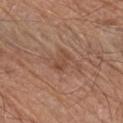image source: ~15 mm crop, total-body skin-cancer survey; body site: the arm; subject: male, approximately 70 years of age; image-analysis metrics: an automated nevus-likeness rating near 0 out of 100 and a lesion-detection confidence of about 100/100; size: ≈3 mm; tile lighting: white-light.